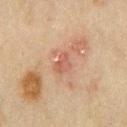{
  "site": "chest",
  "lighting": "cross-polarized",
  "lesion_size": {
    "long_diameter_mm_approx": 2.5
  },
  "image": {
    "source": "total-body photography crop",
    "field_of_view_mm": 15
  },
  "patient": {
    "sex": "male",
    "age_approx": 70
  }
}